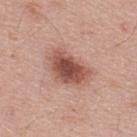Cropped from a whole-body photographic skin survey; the tile spans about 15 mm. The patient is a male aged around 45. The lesion is located on the upper back. Measured at roughly 5.5 mm in maximum diameter. Imaged with white-light lighting.